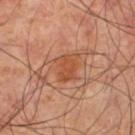The lesion was photographed on a routine skin check and not biopsied; there is no pathology result.
An algorithmic analysis of the crop reported an average lesion color of about L≈49 a*≈26 b*≈35 (CIELAB) and a normalized border contrast of about 6.5. The software also gave border irregularity of about 3.5 on a 0–10 scale, a within-lesion color-variation index near 1.5/10, and peripheral color asymmetry of about 0.5. The analysis additionally found an automated nevus-likeness rating near 15 out of 100 and a lesion-detection confidence of about 100/100.
Located on the left thigh.
A male subject about 60 years old.
Longest diameter approximately 3 mm.
This is a cross-polarized tile.
Cropped from a whole-body photographic skin survey; the tile spans about 15 mm.